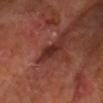<tbp_lesion>
<biopsy_status>not biopsied; imaged during a skin examination</biopsy_status>
<automated_metrics>
  <area_mm2_approx>4.0</area_mm2_approx>
  <eccentricity>0.9</eccentricity>
  <cielab_L>29</cielab_L>
  <cielab_a>25</cielab_a>
  <cielab_b>25</cielab_b>
  <vs_skin_contrast_norm>9.0</vs_skin_contrast_norm>
  <border_irregularity_0_10>3.5</border_irregularity_0_10>
  <peripheral_color_asymmetry>0.5</peripheral_color_asymmetry>
</automated_metrics>
<patient>
  <sex>male</sex>
  <age_approx>65</age_approx>
</patient>
<lesion_size>
  <long_diameter_mm_approx>3.5</long_diameter_mm_approx>
</lesion_size>
<lighting>cross-polarized</lighting>
<image>
  <source>total-body photography crop</source>
  <field_of_view_mm>15</field_of_view_mm>
</image>
<site>head or neck</site>
</tbp_lesion>